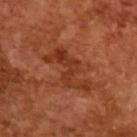Recorded during total-body skin imaging; not selected for excision or biopsy. A male patient in their mid- to late 60s. This image is a 15 mm lesion crop taken from a total-body photograph.